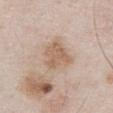This is a white-light tile.
A 15 mm close-up extracted from a 3D total-body photography capture.
Measured at roughly 4 mm in maximum diameter.
On the chest.
A male patient roughly 55 years of age.